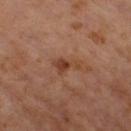The lesion was photographed on a routine skin check and not biopsied; there is no pathology result. A male patient, aged approximately 60. Cropped from a total-body skin-imaging series; the visible field is about 15 mm. Located on the right thigh.